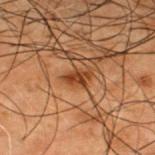Findings:
- biopsy status: catalogued during a skin exam; not biopsied
- lesion diameter: ~2.5 mm (longest diameter)
- anatomic site: the upper back
- lighting: cross-polarized
- image source: ~15 mm crop, total-body skin-cancer survey
- patient: male, aged around 50
- TBP lesion metrics: an area of roughly 3 mm² and a symmetry-axis asymmetry near 0.3; a within-lesion color-variation index near 1.5/10 and peripheral color asymmetry of about 0.5; a classifier nevus-likeness of about 80/100